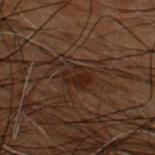{
  "biopsy_status": "not biopsied; imaged during a skin examination",
  "image": {
    "source": "total-body photography crop",
    "field_of_view_mm": 15
  },
  "site": "upper back",
  "patient": {
    "sex": "male",
    "age_approx": 50
  }
}